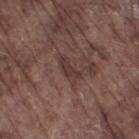biopsy status: imaged on a skin check; not biopsied | image-analysis metrics: an eccentricity of roughly 0.9 and two-axis asymmetry of about 0.4 | diameter: ~3.5 mm (longest diameter) | image: total-body-photography crop, ~15 mm field of view | anatomic site: the right thigh | illumination: white-light illumination | patient: male, approximately 75 years of age.